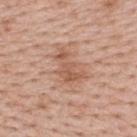workup: catalogued during a skin exam; not biopsied
subject: male, aged 38 to 42
tile lighting: white-light illumination
location: the upper back
image: 15 mm crop, total-body photography
automated lesion analysis: a lesion area of about 7 mm², a shape eccentricity near 0.85, and a symmetry-axis asymmetry near 0.55; a classifier nevus-likeness of about 10/100 and lesion-presence confidence of about 100/100